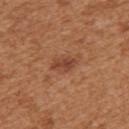Captured during whole-body skin photography for melanoma surveillance; the lesion was not biopsied.
From the upper back.
A female patient, roughly 40 years of age.
Cropped from a whole-body photographic skin survey; the tile spans about 15 mm.